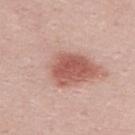image source: 15 mm crop, total-body photography
tile lighting: white-light illumination
subject: male, about 25 years old
site: the upper back